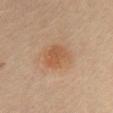Captured during whole-body skin photography for melanoma surveillance; the lesion was not biopsied. Cropped from a total-body skin-imaging series; the visible field is about 15 mm. Located on the chest. Longest diameter approximately 3.5 mm. A female subject, roughly 60 years of age.